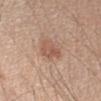| field | value |
|---|---|
| workup | catalogued during a skin exam; not biopsied |
| automated lesion analysis | a footprint of about 5 mm² and two-axis asymmetry of about 0.35; a border-irregularity index near 3.5/10, a color-variation rating of about 3.5/10, and peripheral color asymmetry of about 1 |
| subject | male, aged 33 to 37 |
| image | ~15 mm tile from a whole-body skin photo |
| anatomic site | the right upper arm |
| lighting | white-light illumination |
| lesion size | ≈3.5 mm |